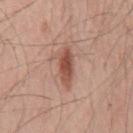  biopsy_status: not biopsied; imaged during a skin examination
  image:
    source: total-body photography crop
    field_of_view_mm: 15
  patient:
    sex: male
    age_approx: 55
  site: front of the torso